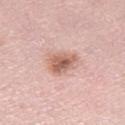Captured during whole-body skin photography for melanoma surveillance; the lesion was not biopsied. This is a white-light tile. A region of skin cropped from a whole-body photographic capture, roughly 15 mm wide. From the leg. The patient is a female about 65 years old.